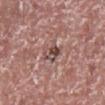Q: How was this image acquired?
A: total-body-photography crop, ~15 mm field of view
Q: Who is the patient?
A: male, roughly 75 years of age
Q: Illumination type?
A: white-light illumination
Q: Automated lesion metrics?
A: a lesion area of about 5 mm², an outline eccentricity of about 0.8 (0 = round, 1 = elongated), and a symmetry-axis asymmetry near 0.25; an average lesion color of about L≈47 a*≈19 b*≈21 (CIELAB), roughly 11 lightness units darker than nearby skin, and a normalized border contrast of about 8; a border-irregularity rating of about 2.5/10, a color-variation rating of about 8.5/10, and peripheral color asymmetry of about 3.5
Q: What is the anatomic site?
A: the right lower leg